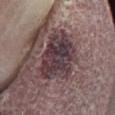Q: Was this lesion biopsied?
A: total-body-photography surveillance lesion; no biopsy
Q: What is the imaging modality?
A: 15 mm crop, total-body photography
Q: Who is the patient?
A: male, aged 73–77
Q: Lesion size?
A: about 7.5 mm
Q: Where on the body is the lesion?
A: the left lower leg